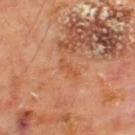The lesion was tiled from a total-body skin photograph and was not biopsied.
A male patient aged approximately 65.
A 15 mm close-up tile from a total-body photography series done for melanoma screening.
An algorithmic analysis of the crop reported an area of roughly 2.5 mm² and two-axis asymmetry of about 0.45. The software also gave border irregularity of about 5 on a 0–10 scale, internal color variation of about 0 on a 0–10 scale, and peripheral color asymmetry of about 0. The software also gave a nevus-likeness score of about 0/100 and a detector confidence of about 100 out of 100 that the crop contains a lesion.
Located on the upper back.
Approximately 2.5 mm at its widest.